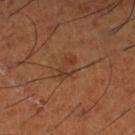Q: Was a biopsy performed?
A: catalogued during a skin exam; not biopsied
Q: What are the patient's age and sex?
A: male, roughly 65 years of age
Q: Automated lesion metrics?
A: about 6 CIELAB-L* units darker than the surrounding skin and a lesion-to-skin contrast of about 5.5 (normalized; higher = more distinct); a classifier nevus-likeness of about 5/100 and lesion-presence confidence of about 100/100
Q: What is the lesion's diameter?
A: about 3 mm
Q: Lesion location?
A: the left lower leg
Q: What is the imaging modality?
A: ~15 mm tile from a whole-body skin photo
Q: Illumination type?
A: cross-polarized illumination